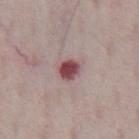biopsy status = imaged on a skin check; not biopsied
patient = male, aged approximately 70
tile lighting = white-light illumination
site = the abdomen
image source = ~15 mm tile from a whole-body skin photo
automated lesion analysis = a lesion area of about 4.5 mm², an eccentricity of roughly 0.5, and a symmetry-axis asymmetry near 0.2; a lesion color around L≈46 a*≈26 b*≈16 in CIELAB and about 17 CIELAB-L* units darker than the surrounding skin; a nevus-likeness score of about 0/100